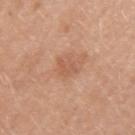Imaged during a routine full-body skin examination; the lesion was not biopsied and no histopathology is available. Captured under white-light illumination. A lesion tile, about 15 mm wide, cut from a 3D total-body photograph. The recorded lesion diameter is about 2.5 mm. The patient is a female aged 38 to 42. The lesion is located on the left upper arm.